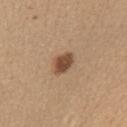- biopsy status: no biopsy performed (imaged during a skin exam)
- image-analysis metrics: a lesion color around L≈48 a*≈19 b*≈31 in CIELAB and roughly 14 lightness units darker than nearby skin; a nevus-likeness score of about 95/100 and a detector confidence of about 100 out of 100 that the crop contains a lesion
- subject: female, aged 58 to 62
- illumination: white-light illumination
- lesion size: ~3 mm (longest diameter)
- body site: the left upper arm
- imaging modality: total-body-photography crop, ~15 mm field of view